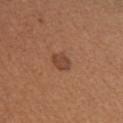Impression: The lesion was tiled from a total-body skin photograph and was not biopsied.